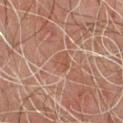This is a cross-polarized tile. Approximately 2.5 mm at its widest. A male subject aged 43 to 47. Cropped from a total-body skin-imaging series; the visible field is about 15 mm. Located on the chest.